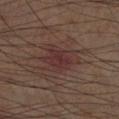Q: Is there a histopathology result?
A: no biopsy performed (imaged during a skin exam)
Q: What is the anatomic site?
A: the leg
Q: Patient demographics?
A: male, about 60 years old
Q: What kind of image is this?
A: 15 mm crop, total-body photography